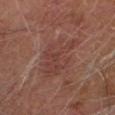Located on the head or neck.
A subject in their mid- to late 60s.
This image is a 15 mm lesion crop taken from a total-body photograph.
This is a cross-polarized tile.
Longest diameter approximately 5.5 mm.